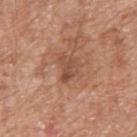Impression: Recorded during total-body skin imaging; not selected for excision or biopsy. Image and clinical context: Cropped from a total-body skin-imaging series; the visible field is about 15 mm. On the upper back. This is a white-light tile. A male patient roughly 65 years of age. The recorded lesion diameter is about 3 mm.